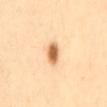Image and clinical context: The tile uses cross-polarized illumination. Cropped from a total-body skin-imaging series; the visible field is about 15 mm. Longest diameter approximately 3 mm. The lesion-visualizer software estimated a classifier nevus-likeness of about 100/100 and a lesion-detection confidence of about 100/100. From the back. A female patient, roughly 40 years of age.